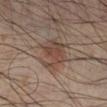lesion diameter=about 4.5 mm; patient=male, aged 43–47; body site=the right lower leg; imaging modality=total-body-photography crop, ~15 mm field of view.